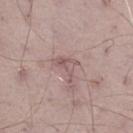This lesion was catalogued during total-body skin photography and was not selected for biopsy. Approximately 3.5 mm at its widest. A roughly 15 mm field-of-view crop from a total-body skin photograph. The tile uses white-light illumination. From the right thigh. A male patient, aged approximately 70.